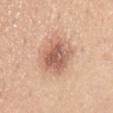Recorded during total-body skin imaging; not selected for excision or biopsy. Longest diameter approximately 5 mm. A region of skin cropped from a whole-body photographic capture, roughly 15 mm wide. The lesion is located on the lower back. This is a white-light tile. A male patient, in their 50s.